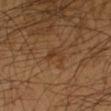| key | value |
|---|---|
| image source | total-body-photography crop, ~15 mm field of view |
| body site | the arm |
| lesion diameter | ≈3 mm |
| patient | male, aged 63–67 |
| illumination | cross-polarized illumination |